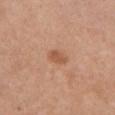<lesion>
  <biopsy_status>not biopsied; imaged during a skin examination</biopsy_status>
  <automated_metrics>
    <area_mm2_approx>3.5</area_mm2_approx>
    <eccentricity>0.8</eccentricity>
    <shape_asymmetry>0.3</shape_asymmetry>
    <color_variation_0_10>1.5</color_variation_0_10>
    <peripheral_color_asymmetry>0.5</peripheral_color_asymmetry>
    <nevus_likeness_0_100>20</nevus_likeness_0_100>
    <lesion_detection_confidence_0_100>100</lesion_detection_confidence_0_100>
  </automated_metrics>
  <site>front of the torso</site>
  <lesion_size>
    <long_diameter_mm_approx>2.5</long_diameter_mm_approx>
  </lesion_size>
  <patient>
    <sex>female</sex>
    <age_approx>55</age_approx>
  </patient>
  <image>
    <source>total-body photography crop</source>
    <field_of_view_mm>15</field_of_view_mm>
  </image>
  <lighting>white-light</lighting>
</lesion>